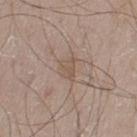Notes:
• subject: male, aged around 65
• acquisition: total-body-photography crop, ~15 mm field of view
• illumination: white-light illumination
• lesion diameter: ~3 mm (longest diameter)
• body site: the left upper arm